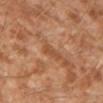Assessment: No biopsy was performed on this lesion — it was imaged during a full skin examination and was not determined to be concerning. Clinical summary: The recorded lesion diameter is about 2.5 mm. The lesion is on the leg. A 15 mm close-up tile from a total-body photography series done for melanoma screening. The patient is a male approximately 30 years of age. The total-body-photography lesion software estimated a within-lesion color-variation index near 0.5/10.